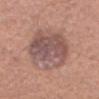<tbp_lesion>
  <biopsy_status>not biopsied; imaged during a skin examination</biopsy_status>
  <site>head or neck</site>
  <automated_metrics>
    <area_mm2_approx>29.0</area_mm2_approx>
    <eccentricity>0.6</eccentricity>
    <shape_asymmetry>0.15</shape_asymmetry>
    <cielab_L>52</cielab_L>
    <cielab_a>19</cielab_a>
    <cielab_b>22</cielab_b>
    <vs_skin_darker_L>10.0</vs_skin_darker_L>
    <vs_skin_contrast_norm>8.0</vs_skin_contrast_norm>
    <border_irregularity_0_10>2.5</border_irregularity_0_10>
    <color_variation_0_10>6.0</color_variation_0_10>
    <peripheral_color_asymmetry>2.0</peripheral_color_asymmetry>
  </automated_metrics>
  <image>
    <source>total-body photography crop</source>
    <field_of_view_mm>15</field_of_view_mm>
  </image>
  <patient>
    <sex>female</sex>
    <age_approx>40</age_approx>
  </patient>
  <lighting>white-light</lighting>
</tbp_lesion>